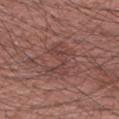  patient:
    sex: male
    age_approx: 60
  automated_metrics:
    cielab_L: 42
    cielab_a: 22
    cielab_b: 22
    vs_skin_darker_L: 6.0
    vs_skin_contrast_norm: 5.0
    border_irregularity_0_10: 8.5
    color_variation_0_10: 2.5
    peripheral_color_asymmetry: 0.5
    nevus_likeness_0_100: 0
  image:
    source: total-body photography crop
    field_of_view_mm: 15
  site: right forearm
  lighting: white-light